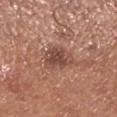Image and clinical context:
Approximately 4 mm at its widest. This image is a 15 mm lesion crop taken from a total-body photograph. Imaged with white-light lighting. The patient is a male aged 68–72. On the right lower leg.